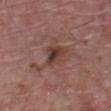This lesion was catalogued during total-body skin photography and was not selected for biopsy. Automated image analysis of the tile measured a border-irregularity rating of about 2.5/10, a color-variation rating of about 6/10, and a peripheral color-asymmetry measure near 2. The software also gave an automated nevus-likeness rating near 25 out of 100 and a detector confidence of about 100 out of 100 that the crop contains a lesion. A 15 mm close-up tile from a total-body photography series done for melanoma screening. The lesion is located on the chest. The patient is a male aged around 65.